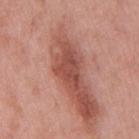Impression:
Captured during whole-body skin photography for melanoma surveillance; the lesion was not biopsied.
Context:
The tile uses white-light illumination. A 15 mm crop from a total-body photograph taken for skin-cancer surveillance. Longest diameter approximately 4.5 mm. The patient is a male in their mid- to late 50s. The lesion is on the mid back.